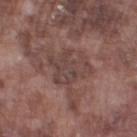Q: Is there a histopathology result?
A: no biopsy performed (imaged during a skin exam)
Q: What are the patient's age and sex?
A: male, about 75 years old
Q: Illumination type?
A: white-light
Q: Automated lesion metrics?
A: a nevus-likeness score of about 0/100 and a detector confidence of about 70 out of 100 that the crop contains a lesion
Q: What is the anatomic site?
A: the leg
Q: How was this image acquired?
A: ~15 mm crop, total-body skin-cancer survey